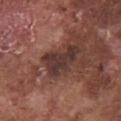Background:
An algorithmic analysis of the crop reported a footprint of about 10 mm². Cropped from a whole-body photographic skin survey; the tile spans about 15 mm. A male subject, approximately 75 years of age. On the chest. Approximately 5 mm at its widest. The tile uses white-light illumination.